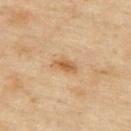Recorded during total-body skin imaging; not selected for excision or biopsy.
A male patient in their mid- to late 40s.
From the upper back.
A 15 mm close-up extracted from a 3D total-body photography capture.
Approximately 3 mm at its widest.